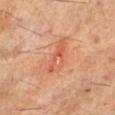biopsy status = catalogued during a skin exam; not biopsied | site = the left lower leg | imaging modality = ~15 mm tile from a whole-body skin photo | lesion size = about 5 mm | patient = male, aged 58–62.